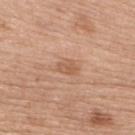Imaged during a routine full-body skin examination; the lesion was not biopsied and no histopathology is available. Approximately 2.5 mm at its widest. This is a white-light tile. A male patient aged around 70. A lesion tile, about 15 mm wide, cut from a 3D total-body photograph. An algorithmic analysis of the crop reported a nevus-likeness score of about 0/100 and lesion-presence confidence of about 100/100. The lesion is on the upper back.